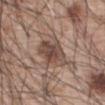Captured during whole-body skin photography for melanoma surveillance; the lesion was not biopsied. Cropped from a whole-body photographic skin survey; the tile spans about 15 mm. The total-body-photography lesion software estimated a lesion area of about 11 mm², a shape eccentricity near 0.7, and a symmetry-axis asymmetry near 0.15. The software also gave a lesion-to-skin contrast of about 8 (normalized; higher = more distinct). The analysis additionally found a border-irregularity index near 2/10 and radial color variation of about 2. On the abdomen. Captured under white-light illumination. The subject is a male about 60 years old.